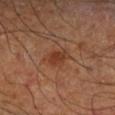Imaged with cross-polarized lighting.
Automated image analysis of the tile measured an area of roughly 5.5 mm² and a symmetry-axis asymmetry near 0.3.
A male subject in their mid-60s.
The lesion is on the right lower leg.
The recorded lesion diameter is about 3 mm.
Cropped from a total-body skin-imaging series; the visible field is about 15 mm.